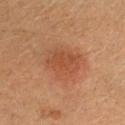Assessment:
Part of a total-body skin-imaging series; this lesion was reviewed on a skin check and was not flagged for biopsy.
Context:
Imaged with cross-polarized lighting. A region of skin cropped from a whole-body photographic capture, roughly 15 mm wide. Located on the head or neck. The patient is a male approximately 40 years of age.